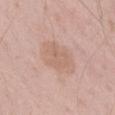{
  "lighting": "white-light",
  "patient": {
    "sex": "male",
    "age_approx": 55
  },
  "image": {
    "source": "total-body photography crop",
    "field_of_view_mm": 15
  },
  "site": "right thigh",
  "automated_metrics": {
    "area_mm2_approx": 15.0,
    "eccentricity": 0.6,
    "shape_asymmetry": 0.15,
    "cielab_L": 63,
    "cielab_a": 18,
    "cielab_b": 27,
    "vs_skin_darker_L": 6.0,
    "vs_skin_contrast_norm": 5.0
  }
}